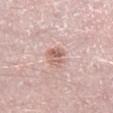* follow-up — imaged on a skin check; not biopsied
* lesion diameter — ~3 mm (longest diameter)
* location — the right lower leg
* image — ~15 mm tile from a whole-body skin photo
* tile lighting — white-light
* patient — male, aged 48–52
* automated metrics — a footprint of about 5 mm² and an outline eccentricity of about 0.7 (0 = round, 1 = elongated); a mean CIELAB color near L≈62 a*≈21 b*≈25, a lesion–skin lightness drop of about 11, and a lesion-to-skin contrast of about 7 (normalized; higher = more distinct)